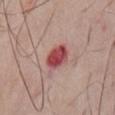Case summary:
• notes — total-body-photography surveillance lesion; no biopsy